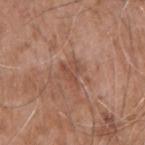Q: What is the imaging modality?
A: ~15 mm tile from a whole-body skin photo
Q: Lesion location?
A: the right upper arm
Q: Patient demographics?
A: male, in their mid- to late 70s